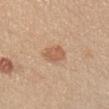  biopsy_status: not biopsied; imaged during a skin examination
  image:
    source: total-body photography crop
    field_of_view_mm: 15
  automated_metrics:
    nevus_likeness_0_100: 80
    lesion_detection_confidence_0_100: 100
  site: chest
  patient:
    sex: female
    age_approx: 45
  lighting: white-light